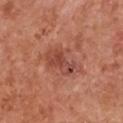Clinical impression:
The lesion was tiled from a total-body skin photograph and was not biopsied.
Clinical summary:
Captured under white-light illumination. The lesion is on the chest. The lesion's longest dimension is about 5 mm. This image is a 15 mm lesion crop taken from a total-body photograph. A female subject, aged around 50.